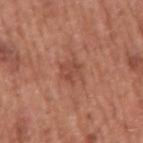The lesion was photographed on a routine skin check and not biopsied; there is no pathology result.
Imaged with white-light lighting.
Automated image analysis of the tile measured a lesion area of about 5 mm², an eccentricity of roughly 0.45, and a shape-asymmetry score of about 0.35 (0 = symmetric). And it measured an average lesion color of about L≈48 a*≈25 b*≈29 (CIELAB) and a normalized border contrast of about 5.5. The software also gave border irregularity of about 4.5 on a 0–10 scale and peripheral color asymmetry of about 1.
A male patient approximately 75 years of age.
A 15 mm crop from a total-body photograph taken for skin-cancer surveillance.
Located on the right upper arm.
Longest diameter approximately 3 mm.